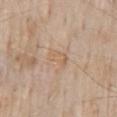  biopsy_status: not biopsied; imaged during a skin examination
  image:
    source: total-body photography crop
    field_of_view_mm: 15
  lighting: white-light
  site: mid back
  lesion_size:
    long_diameter_mm_approx: 3.0
  patient:
    sex: male
    age_approx: 60
  automated_metrics:
    border_irregularity_0_10: 3.0
    peripheral_color_asymmetry: 1.0
    lesion_detection_confidence_0_100: 100